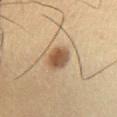{"biopsy_status": "not biopsied; imaged during a skin examination", "site": "left lower leg", "automated_metrics": {"eccentricity": 0.65, "shape_asymmetry": 0.1, "cielab_L": 47, "cielab_a": 16, "cielab_b": 29, "vs_skin_darker_L": 13.0, "vs_skin_contrast_norm": 9.5, "nevus_likeness_0_100": 95, "lesion_detection_confidence_0_100": 100}, "lighting": "cross-polarized", "image": {"source": "total-body photography crop", "field_of_view_mm": 15}, "patient": {"sex": "male", "age_approx": 35}, "lesion_size": {"long_diameter_mm_approx": 3.5}}